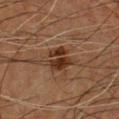  biopsy_status: not biopsied; imaged during a skin examination
  site: front of the torso
  image:
    source: total-body photography crop
    field_of_view_mm: 15
  lesion_size:
    long_diameter_mm_approx: 3.0
  patient:
    sex: male
    age_approx: 65